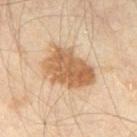Case summary:
- notes — catalogued during a skin exam; not biopsied
- lighting — cross-polarized
- location — the left thigh
- lesion diameter — about 6.5 mm
- image source — 15 mm crop, total-body photography
- subject — aged around 55